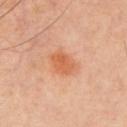{"biopsy_status": "not biopsied; imaged during a skin examination", "patient": {"sex": "male", "age_approx": 50}, "site": "chest", "lighting": "cross-polarized", "image": {"source": "total-body photography crop", "field_of_view_mm": 15}, "lesion_size": {"long_diameter_mm_approx": 3.5}, "automated_metrics": {"cielab_L": 61, "cielab_a": 28, "cielab_b": 39, "vs_skin_darker_L": 9.0, "vs_skin_contrast_norm": 7.0, "nevus_likeness_0_100": 90, "lesion_detection_confidence_0_100": 100}}